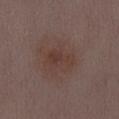The lesion was tiled from a total-body skin photograph and was not biopsied. Imaged with white-light lighting. Automated image analysis of the tile measured a border-irregularity rating of about 2.5/10, a within-lesion color-variation index near 3.5/10, and peripheral color asymmetry of about 1. And it measured an automated nevus-likeness rating near 15 out of 100 and a detector confidence of about 100 out of 100 that the crop contains a lesion. A roughly 15 mm field-of-view crop from a total-body skin photograph. The lesion is located on the abdomen. The lesion's longest dimension is about 4 mm. A female subject, about 30 years old.